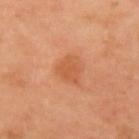Clinical impression:
This lesion was catalogued during total-body skin photography and was not selected for biopsy.
Clinical summary:
From the left upper arm. A female patient in their mid-50s. A 15 mm close-up extracted from a 3D total-body photography capture.